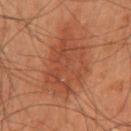* notes · catalogued during a skin exam; not biopsied
* illumination · cross-polarized illumination
* subject · male, aged 58–62
* anatomic site · the mid back
* image · 15 mm crop, total-body photography
* automated metrics · a lesion color around L≈35 a*≈21 b*≈27 in CIELAB and a normalized border contrast of about 6; a within-lesion color-variation index near 3/10 and a peripheral color-asymmetry measure near 1
* lesion diameter · ~7.5 mm (longest diameter)